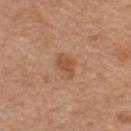Part of a total-body skin-imaging series; this lesion was reviewed on a skin check and was not flagged for biopsy. A roughly 15 mm field-of-view crop from a total-body skin photograph. Approximately 3 mm at its widest. A male subject in their mid- to late 60s. Automated tile analysis of the lesion measured a footprint of about 4.5 mm², a shape eccentricity near 0.8, and a symmetry-axis asymmetry near 0.2. The software also gave a mean CIELAB color near L≈51 a*≈23 b*≈33, roughly 9 lightness units darker than nearby skin, and a normalized lesion–skin contrast near 6.5. And it measured border irregularity of about 2 on a 0–10 scale, a within-lesion color-variation index near 2/10, and a peripheral color-asymmetry measure near 0.5. And it measured a detector confidence of about 100 out of 100 that the crop contains a lesion. Located on the chest. Imaged with white-light lighting.